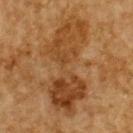Notes:
• notes — no biopsy performed (imaged during a skin exam)
• illumination — cross-polarized
• lesion size — ≈11.5 mm
• image — ~15 mm crop, total-body skin-cancer survey
• subject — male, approximately 85 years of age or older
• site — the upper back